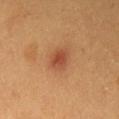About 2.5 mm across. A 15 mm crop from a total-body photograph taken for skin-cancer surveillance. Imaged with cross-polarized lighting. On the chest. The lesion-visualizer software estimated an eccentricity of roughly 0.65 and a shape-asymmetry score of about 0.2 (0 = symmetric). It also reported a border-irregularity rating of about 1.5/10 and a peripheral color-asymmetry measure near 0.5. A female subject aged around 40.